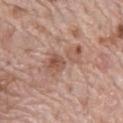biopsy status: no biopsy performed (imaged during a skin exam)
image source: ~15 mm tile from a whole-body skin photo
subject: male, about 70 years old
illumination: white-light
anatomic site: the mid back
lesion diameter: about 6.5 mm
image-analysis metrics: an average lesion color of about L≈55 a*≈20 b*≈27 (CIELAB), about 9 CIELAB-L* units darker than the surrounding skin, and a lesion-to-skin contrast of about 6 (normalized; higher = more distinct)